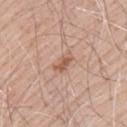workup = no biopsy performed (imaged during a skin exam)
body site = the upper back
imaging modality = total-body-photography crop, ~15 mm field of view
patient = male, aged around 70